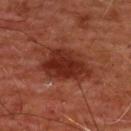A roughly 15 mm field-of-view crop from a total-body skin photograph.
An algorithmic analysis of the crop reported two-axis asymmetry of about 0.25. The analysis additionally found an average lesion color of about L≈27 a*≈26 b*≈27 (CIELAB), about 10 CIELAB-L* units darker than the surrounding skin, and a lesion-to-skin contrast of about 9.5 (normalized; higher = more distinct). The analysis additionally found an automated nevus-likeness rating near 70 out of 100 and a detector confidence of about 100 out of 100 that the crop contains a lesion.
About 6 mm across.
The patient is a male aged 58–62.
From the front of the torso.